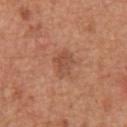Clinical impression:
No biopsy was performed on this lesion — it was imaged during a full skin examination and was not determined to be concerning.
Acquisition and patient details:
Imaged with white-light lighting. The lesion-visualizer software estimated a mean CIELAB color near L≈50 a*≈24 b*≈32, a lesion–skin lightness drop of about 8, and a normalized lesion–skin contrast near 5.5. The software also gave internal color variation of about 2 on a 0–10 scale and peripheral color asymmetry of about 0.5. A close-up tile cropped from a whole-body skin photograph, about 15 mm across. Approximately 3 mm at its widest. The subject is a male about 65 years old. From the abdomen.